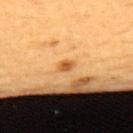{"biopsy_status": "not biopsied; imaged during a skin examination", "site": "upper back", "automated_metrics": {"border_irregularity_0_10": 2.5, "color_variation_0_10": 0.0, "peripheral_color_asymmetry": 0.0}, "patient": {"sex": "female", "age_approx": 65}, "image": {"source": "total-body photography crop", "field_of_view_mm": 15}, "lesion_size": {"long_diameter_mm_approx": 1.5}}